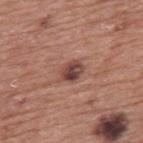workup — imaged on a skin check; not biopsied
subject — male, roughly 75 years of age
automated metrics — a footprint of about 5 mm² and a symmetry-axis asymmetry near 0.2; a mean CIELAB color near L≈44 a*≈22 b*≈24 and a lesion-to-skin contrast of about 10 (normalized; higher = more distinct); an automated nevus-likeness rating near 80 out of 100 and a lesion-detection confidence of about 100/100
lighting — white-light illumination
lesion diameter — about 3 mm
anatomic site — the back
imaging modality — 15 mm crop, total-body photography